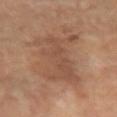Findings:
• patient — female, aged around 70
• site — the left forearm
• lesion diameter — ≈9 mm
• tile lighting — cross-polarized illumination
• image source — ~15 mm crop, total-body skin-cancer survey